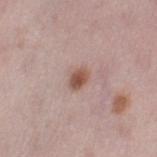{"biopsy_status": "not biopsied; imaged during a skin examination", "image": {"source": "total-body photography crop", "field_of_view_mm": 15}, "site": "left thigh", "lesion_size": {"long_diameter_mm_approx": 2.5}, "patient": {"sex": "female", "age_approx": 50}}